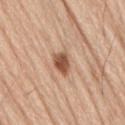The lesion was photographed on a routine skin check and not biopsied; there is no pathology result. A 15 mm close-up extracted from a 3D total-body photography capture. Measured at roughly 3 mm in maximum diameter. An algorithmic analysis of the crop reported a lesion color around L≈54 a*≈22 b*≈32 in CIELAB and a normalized border contrast of about 10. The lesion is located on the lower back. The subject is a male aged 68 to 72. This is a white-light tile.